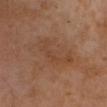Q: Was a biopsy performed?
A: total-body-photography surveillance lesion; no biopsy
Q: What lighting was used for the tile?
A: cross-polarized illumination
Q: What is the lesion's diameter?
A: ~6 mm (longest diameter)
Q: Patient demographics?
A: female, in their mid-50s
Q: What is the anatomic site?
A: the head or neck
Q: What is the imaging modality?
A: total-body-photography crop, ~15 mm field of view